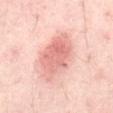{
  "biopsy_status": "not biopsied; imaged during a skin examination",
  "patient": {
    "age_approx": 55
  },
  "image": {
    "source": "total-body photography crop",
    "field_of_view_mm": 15
  },
  "lesion_size": {
    "long_diameter_mm_approx": 6.5
  },
  "site": "abdomen"
}